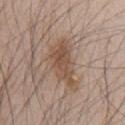lesion size = ~5.5 mm (longest diameter)
acquisition = total-body-photography crop, ~15 mm field of view
lighting = white-light illumination
patient = male, aged around 45
anatomic site = the chest